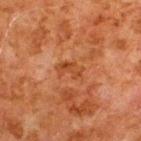Findings:
- image: ~15 mm crop, total-body skin-cancer survey
- patient: male, aged 78 to 82
- anatomic site: the upper back